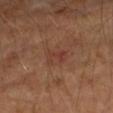Q: Was this lesion biopsied?
A: no biopsy performed (imaged during a skin exam)
Q: What did automated image analysis measure?
A: a lesion color around L≈39 a*≈23 b*≈28 in CIELAB, about 6 CIELAB-L* units darker than the surrounding skin, and a lesion-to-skin contrast of about 5 (normalized; higher = more distinct); border irregularity of about 3 on a 0–10 scale and a color-variation rating of about 2.5/10; a nevus-likeness score of about 0/100 and a detector confidence of about 100 out of 100 that the crop contains a lesion
Q: What are the patient's age and sex?
A: female, aged approximately 60
Q: How was this image acquired?
A: ~15 mm tile from a whole-body skin photo
Q: How large is the lesion?
A: about 2.5 mm
Q: What is the anatomic site?
A: the left forearm